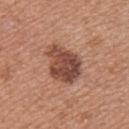Assessment:
Recorded during total-body skin imaging; not selected for excision or biopsy.
Background:
A female patient, aged approximately 35. A region of skin cropped from a whole-body photographic capture, roughly 15 mm wide. From the upper back.